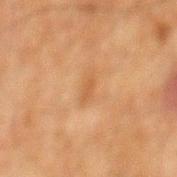Part of a total-body skin-imaging series; this lesion was reviewed on a skin check and was not flagged for biopsy. A lesion tile, about 15 mm wide, cut from a 3D total-body photograph. A male patient, in their 60s. Located on the mid back. Measured at roughly 3 mm in maximum diameter.